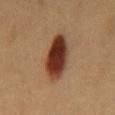The lesion was photographed on a routine skin check and not biopsied; there is no pathology result. The patient is a female aged 38–42. Measured at roughly 6 mm in maximum diameter. A close-up tile cropped from a whole-body skin photograph, about 15 mm across. Captured under cross-polarized illumination. On the abdomen.